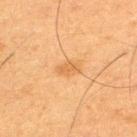subject: male, aged approximately 35; automated lesion analysis: an automated nevus-likeness rating near 10 out of 100; lesion diameter: ~2.5 mm (longest diameter); lighting: cross-polarized; site: the upper back; imaging modality: total-body-photography crop, ~15 mm field of view.